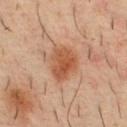No biopsy was performed on this lesion — it was imaged during a full skin examination and was not determined to be concerning. Measured at roughly 4.5 mm in maximum diameter. On the chest. Captured under cross-polarized illumination. Cropped from a whole-body photographic skin survey; the tile spans about 15 mm. A male patient aged around 35.